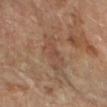Recorded during total-body skin imaging; not selected for excision or biopsy.
A female subject, aged 58 to 62.
Approximately 4.5 mm at its widest.
Located on the arm.
This image is a 15 mm lesion crop taken from a total-body photograph.